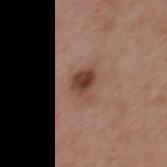| field | value |
|---|---|
| workup | catalogued during a skin exam; not biopsied |
| subject | male, roughly 55 years of age |
| automated metrics | an area of roughly 6 mm² and a shape-asymmetry score of about 0.3 (0 = symmetric); a border-irregularity rating of about 3/10 and a within-lesion color-variation index near 4.5/10 |
| image | ~15 mm tile from a whole-body skin photo |
| site | the mid back |
| lesion size | about 3 mm |
| tile lighting | white-light |